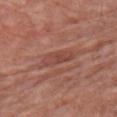follow-up = catalogued during a skin exam; not biopsied
lighting = white-light illumination
acquisition = total-body-photography crop, ~15 mm field of view
site = the right upper arm
patient = male, aged 78–82
automated lesion analysis = a lesion area of about 6 mm² and a shape eccentricity near 0.85; border irregularity of about 2 on a 0–10 scale, a within-lesion color-variation index near 3/10, and a peripheral color-asymmetry measure near 1; a lesion-detection confidence of about 95/100
lesion size = ≈3.5 mm